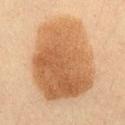Imaged during a routine full-body skin examination; the lesion was not biopsied and no histopathology is available. Located on the chest. The recorded lesion diameter is about 10 mm. A male patient, about 35 years old. A lesion tile, about 15 mm wide, cut from a 3D total-body photograph. Captured under cross-polarized illumination.